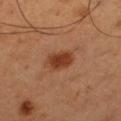Q: Was a biopsy performed?
A: no biopsy performed (imaged during a skin exam)
Q: What kind of image is this?
A: 15 mm crop, total-body photography
Q: What are the patient's age and sex?
A: male, in their 50s
Q: What is the anatomic site?
A: the left thigh
Q: Automated lesion metrics?
A: an area of roughly 7 mm², an outline eccentricity of about 0.7 (0 = round, 1 = elongated), and a symmetry-axis asymmetry near 0.2; a mean CIELAB color near L≈40 a*≈25 b*≈34, a lesion–skin lightness drop of about 11, and a lesion-to-skin contrast of about 9.5 (normalized; higher = more distinct)